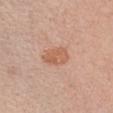| field | value |
|---|---|
| lesion size | ≈3.5 mm |
| site | the chest |
| imaging modality | ~15 mm tile from a whole-body skin photo |
| patient | female, roughly 40 years of age |
| automated metrics | border irregularity of about 2 on a 0–10 scale, a color-variation rating of about 3/10, and peripheral color asymmetry of about 1 |
| tile lighting | white-light illumination |